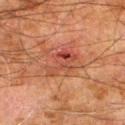Assessment: This lesion was catalogued during total-body skin photography and was not selected for biopsy. Image and clinical context: Imaged with cross-polarized lighting. The subject is a male approximately 80 years of age. The lesion-visualizer software estimated a color-variation rating of about 7/10. The software also gave a classifier nevus-likeness of about 0/100 and a detector confidence of about 100 out of 100 that the crop contains a lesion. A 15 mm crop from a total-body photograph taken for skin-cancer surveillance. Measured at roughly 4.5 mm in maximum diameter. Located on the right thigh.